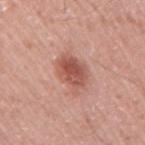<record>
  <biopsy_status>not biopsied; imaged during a skin examination</biopsy_status>
  <lesion_size>
    <long_diameter_mm_approx>4.5</long_diameter_mm_approx>
  </lesion_size>
  <automated_metrics>
    <area_mm2_approx>10.0</area_mm2_approx>
    <eccentricity>0.75</eccentricity>
    <shape_asymmetry>0.2</shape_asymmetry>
    <cielab_L>54</cielab_L>
    <cielab_a>26</cielab_a>
    <cielab_b>28</cielab_b>
    <border_irregularity_0_10>2.5</border_irregularity_0_10>
    <color_variation_0_10>4.5</color_variation_0_10>
    <peripheral_color_asymmetry>1.5</peripheral_color_asymmetry>
  </automated_metrics>
  <patient>
    <sex>male</sex>
    <age_approx>50</age_approx>
  </patient>
  <lighting>white-light</lighting>
  <image>
    <source>total-body photography crop</source>
    <field_of_view_mm>15</field_of_view_mm>
  </image>
  <site>arm</site>
</record>